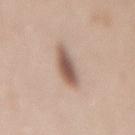Recorded during total-body skin imaging; not selected for excision or biopsy. From the mid back. A female subject, aged 48 to 52. Imaged with white-light lighting. Automated tile analysis of the lesion measured a mean CIELAB color near L≈56 a*≈17 b*≈25 and about 15 CIELAB-L* units darker than the surrounding skin. And it measured a classifier nevus-likeness of about 100/100. A 15 mm crop from a total-body photograph taken for skin-cancer surveillance.